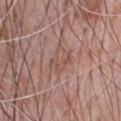{
  "biopsy_status": "not biopsied; imaged during a skin examination",
  "automated_metrics": {
    "cielab_L": 52,
    "cielab_a": 20,
    "cielab_b": 25,
    "vs_skin_darker_L": 6.0,
    "vs_skin_contrast_norm": 4.5,
    "border_irregularity_0_10": 2.5,
    "color_variation_0_10": 4.0,
    "peripheral_color_asymmetry": 1.5
  },
  "lighting": "white-light",
  "patient": {
    "sex": "male",
    "age_approx": 70
  },
  "site": "chest",
  "image": {
    "source": "total-body photography crop",
    "field_of_view_mm": 15
  }
}